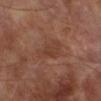{"biopsy_status": "not biopsied; imaged during a skin examination", "image": {"source": "total-body photography crop", "field_of_view_mm": 15}, "lighting": "cross-polarized", "lesion_size": {"long_diameter_mm_approx": 4.5}, "automated_metrics": {"area_mm2_approx": 7.5, "shape_asymmetry": 0.35, "vs_skin_darker_L": 5.0, "vs_skin_contrast_norm": 4.5, "lesion_detection_confidence_0_100": 100}, "site": "right lower leg", "patient": {"sex": "male", "age_approx": 70}}